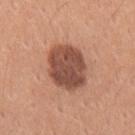biopsy_status: not biopsied; imaged during a skin examination
image:
  source: total-body photography crop
  field_of_view_mm: 15
patient:
  sex: male
  age_approx: 30
site: right upper arm